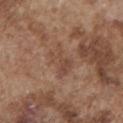Imaged during a routine full-body skin examination; the lesion was not biopsied and no histopathology is available.
Cropped from a total-body skin-imaging series; the visible field is about 15 mm.
Captured under white-light illumination.
A male patient roughly 75 years of age.
From the chest.
Measured at roughly 4 mm in maximum diameter.